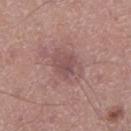The lesion was photographed on a routine skin check and not biopsied; there is no pathology result. Cropped from a whole-body photographic skin survey; the tile spans about 15 mm. The patient is a male aged 53–57. On the right lower leg.